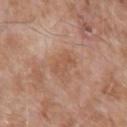Part of a total-body skin-imaging series; this lesion was reviewed on a skin check and was not flagged for biopsy. Captured under white-light illumination. Approximately 3 mm at its widest. The lesion-visualizer software estimated lesion-presence confidence of about 100/100. The lesion is on the left upper arm. A male subject approximately 75 years of age. A 15 mm crop from a total-body photograph taken for skin-cancer surveillance.